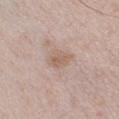Q: Was this lesion biopsied?
A: no biopsy performed (imaged during a skin exam)
Q: Who is the patient?
A: male, in their mid- to late 20s
Q: Illumination type?
A: white-light
Q: Where on the body is the lesion?
A: the chest
Q: How was this image acquired?
A: total-body-photography crop, ~15 mm field of view
Q: What did automated image analysis measure?
A: internal color variation of about 3.5 on a 0–10 scale and peripheral color asymmetry of about 1